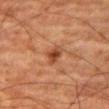follow-up = imaged on a skin check; not biopsied
acquisition = 15 mm crop, total-body photography
body site = the right thigh
subject = male, in their 80s
size = ≈2.5 mm